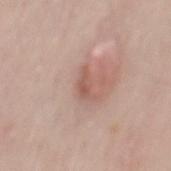Part of a total-body skin-imaging series; this lesion was reviewed on a skin check and was not flagged for biopsy. A 15 mm close-up tile from a total-body photography series done for melanoma screening. A male patient, about 50 years old. Approximately 3 mm at its widest. The lesion is on the mid back. The total-body-photography lesion software estimated a border-irregularity rating of about 3.5/10, a color-variation rating of about 0.5/10, and peripheral color asymmetry of about 0. It also reported a classifier nevus-likeness of about 80/100 and a detector confidence of about 100 out of 100 that the crop contains a lesion.